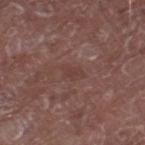The lesion is on the left thigh. The lesion-visualizer software estimated a border-irregularity index near 2/10, internal color variation of about 1 on a 0–10 scale, and peripheral color asymmetry of about 0.5. Cropped from a total-body skin-imaging series; the visible field is about 15 mm. Measured at roughly 2.5 mm in maximum diameter. This is a white-light tile. A male patient, about 65 years old.